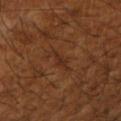<case>
<biopsy_status>not biopsied; imaged during a skin examination</biopsy_status>
<lighting>cross-polarized</lighting>
<lesion_size>
  <long_diameter_mm_approx>4.0</long_diameter_mm_approx>
</lesion_size>
<image>
  <source>total-body photography crop</source>
  <field_of_view_mm>15</field_of_view_mm>
</image>
<site>right upper arm</site>
<automated_metrics>
  <area_mm2_approx>4.0</area_mm2_approx>
  <eccentricity>0.95</eccentricity>
  <shape_asymmetry>0.35</shape_asymmetry>
  <nevus_likeness_0_100>0</nevus_likeness_0_100>
  <lesion_detection_confidence_0_100>65</lesion_detection_confidence_0_100>
</automated_metrics>
<patient>
  <sex>male</sex>
  <age_approx>65</age_approx>
</patient>
</case>